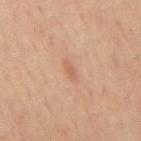Recorded during total-body skin imaging; not selected for excision or biopsy. From the front of the torso. Approximately 2.5 mm at its widest. Automated image analysis of the tile measured a lesion color around L≈48 a*≈19 b*≈26 in CIELAB, roughly 6 lightness units darker than nearby skin, and a normalized border contrast of about 5. The patient is a male aged approximately 70. A 15 mm close-up extracted from a 3D total-body photography capture. Captured under cross-polarized illumination.